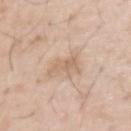  automated_metrics:
    eccentricity: 0.8
    shape_asymmetry: 0.4
    cielab_L: 64
    cielab_a: 16
    cielab_b: 30
    vs_skin_darker_L: 8.0
    nevus_likeness_0_100: 0
    lesion_detection_confidence_0_100: 100
  image:
    source: total-body photography crop
    field_of_view_mm: 15
  patient:
    sex: male
    age_approx: 50
  site: left upper arm